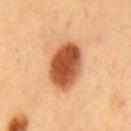Recorded during total-body skin imaging; not selected for excision or biopsy.